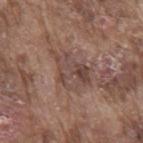This lesion was catalogued during total-body skin photography and was not selected for biopsy.
A roughly 15 mm field-of-view crop from a total-body skin photograph.
Longest diameter approximately 5.5 mm.
The lesion is on the mid back.
A male subject aged 73 to 77.
The total-body-photography lesion software estimated a classifier nevus-likeness of about 0/100 and a lesion-detection confidence of about 85/100.
Captured under white-light illumination.